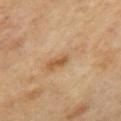{"biopsy_status": "not biopsied; imaged during a skin examination", "site": "mid back", "lesion_size": {"long_diameter_mm_approx": 6.0}, "lighting": "cross-polarized", "image": {"source": "total-body photography crop", "field_of_view_mm": 15}}